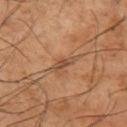workup: imaged on a skin check; not biopsied | acquisition: ~15 mm tile from a whole-body skin photo | lighting: cross-polarized illumination | body site: the leg | patient: male, aged 63 to 67.